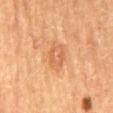| field | value |
|---|---|
| anatomic site | the mid back |
| patient | female, aged 58–62 |
| lighting | cross-polarized |
| lesion size | about 3 mm |
| acquisition | ~15 mm tile from a whole-body skin photo |